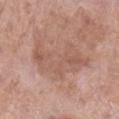– notes — total-body-photography surveillance lesion; no biopsy
– image source — 15 mm crop, total-body photography
– lighting — white-light
– body site — the right lower leg
– patient — female, aged 53–57
– lesion diameter — ~7.5 mm (longest diameter)
– TBP lesion metrics — a lesion area of about 22 mm² and a symmetry-axis asymmetry near 0.4; an average lesion color of about L≈56 a*≈20 b*≈27 (CIELAB), roughly 7 lightness units darker than nearby skin, and a normalized lesion–skin contrast near 5; a border-irregularity index near 6/10, a color-variation rating of about 3.5/10, and a peripheral color-asymmetry measure near 1